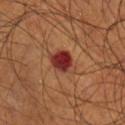Recorded during total-body skin imaging; not selected for excision or biopsy. The subject is a male about 70 years old. Cropped from a whole-body photographic skin survey; the tile spans about 15 mm. Located on the leg.